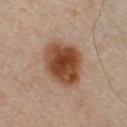Notes:
• workup: imaged on a skin check; not biopsied
• image source: ~15 mm tile from a whole-body skin photo
• lesion diameter: ≈6 mm
• patient: male, aged around 35
• illumination: cross-polarized
• anatomic site: the chest